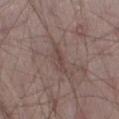Background: Approximately 4 mm at its widest. The total-body-photography lesion software estimated a normalized lesion–skin contrast near 5.5. This image is a 15 mm lesion crop taken from a total-body photograph. This is a white-light tile. Located on the left thigh. The patient is a male about 65 years old.